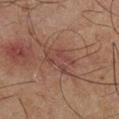Findings:
* biopsy status · total-body-photography surveillance lesion; no biopsy
* acquisition · ~15 mm tile from a whole-body skin photo
* subject · male, in their mid-60s
* site · the leg